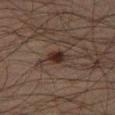Case summary:
• workup: total-body-photography surveillance lesion; no biopsy
• body site: the right thigh
• patient: male, aged 48 to 52
• tile lighting: cross-polarized
• imaging modality: ~15 mm tile from a whole-body skin photo
• diameter: about 2.5 mm
• image-analysis metrics: an outline eccentricity of about 0.65 (0 = round, 1 = elongated) and a shape-asymmetry score of about 0.25 (0 = symmetric); an average lesion color of about L≈21 a*≈12 b*≈17 (CIELAB), about 9 CIELAB-L* units darker than the surrounding skin, and a normalized lesion–skin contrast near 10.5; a border-irregularity index near 2.5/10, a color-variation rating of about 4/10, and radial color variation of about 1.5